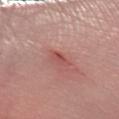No biopsy was performed on this lesion — it was imaged during a full skin examination and was not determined to be concerning.
Located on the right forearm.
A close-up tile cropped from a whole-body skin photograph, about 15 mm across.
A female subject, in their 40s.